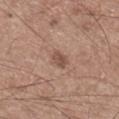Assessment:
The lesion was tiled from a total-body skin photograph and was not biopsied.
Clinical summary:
Automated tile analysis of the lesion measured a border-irregularity rating of about 2.5/10 and radial color variation of about 0. It also reported lesion-presence confidence of about 100/100. Imaged with white-light lighting. A 15 mm close-up tile from a total-body photography series done for melanoma screening. Located on the upper back. The recorded lesion diameter is about 2.5 mm. The subject is a male about 60 years old.